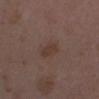Q: What lighting was used for the tile?
A: white-light
Q: What kind of image is this?
A: ~15 mm tile from a whole-body skin photo
Q: Where on the body is the lesion?
A: the right lower leg
Q: How large is the lesion?
A: ≈3 mm
Q: Who is the patient?
A: female, in their mid- to late 30s